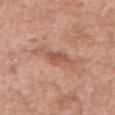No biopsy was performed on this lesion — it was imaged during a full skin examination and was not determined to be concerning.
The recorded lesion diameter is about 6 mm.
A female patient, aged 83 or older.
A 15 mm crop from a total-body photograph taken for skin-cancer surveillance.
The tile uses white-light illumination.
An algorithmic analysis of the crop reported two-axis asymmetry of about 0.4.
From the head or neck.